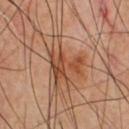Notes:
- follow-up · imaged on a skin check; not biopsied
- diameter · about 6 mm
- illumination · cross-polarized
- patient · male, roughly 60 years of age
- acquisition · 15 mm crop, total-body photography
- location · the chest